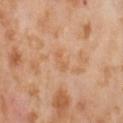follow-up: total-body-photography surveillance lesion; no biopsy | acquisition: 15 mm crop, total-body photography | anatomic site: the abdomen | tile lighting: cross-polarized | patient: female, about 55 years old | image-analysis metrics: a lesion area of about 3.5 mm², an eccentricity of roughly 0.85, and a shape-asymmetry score of about 0.35 (0 = symmetric); an average lesion color of about L≈62 a*≈22 b*≈37 (CIELAB), roughly 5 lightness units darker than nearby skin, and a lesion-to-skin contrast of about 4.5 (normalized; higher = more distinct); border irregularity of about 3.5 on a 0–10 scale, a within-lesion color-variation index near 1/10, and peripheral color asymmetry of about 0.5.